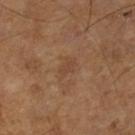A male patient approximately 65 years of age. From the left lower leg. Measured at roughly 3 mm in maximum diameter. The tile uses cross-polarized illumination. A lesion tile, about 15 mm wide, cut from a 3D total-body photograph.